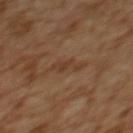Clinical impression: The lesion was tiled from a total-body skin photograph and was not biopsied. Clinical summary: An algorithmic analysis of the crop reported a border-irregularity index near 5/10, a within-lesion color-variation index near 1/10, and a peripheral color-asymmetry measure near 0. A 15 mm close-up extracted from a 3D total-body photography capture. Measured at roughly 4.5 mm in maximum diameter. This is a cross-polarized tile. The patient is a female in their mid-50s. The lesion is located on the upper back.